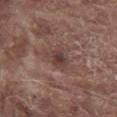notes: imaged on a skin check; not biopsied
lesion size: about 3 mm
patient: male, aged approximately 80
imaging modality: ~15 mm tile from a whole-body skin photo
body site: the chest
illumination: white-light illumination
image-analysis metrics: an area of roughly 6 mm² and two-axis asymmetry of about 0.3; a mean CIELAB color near L≈40 a*≈17 b*≈20 and a normalized lesion–skin contrast near 7